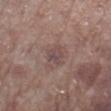Part of a total-body skin-imaging series; this lesion was reviewed on a skin check and was not flagged for biopsy. The lesion is on the right lower leg. This image is a 15 mm lesion crop taken from a total-body photograph. The total-body-photography lesion software estimated a footprint of about 4 mm² and a shape-asymmetry score of about 0.3 (0 = symmetric). The software also gave a lesion color around L≈46 a*≈17 b*≈17 in CIELAB, a lesion–skin lightness drop of about 7, and a lesion-to-skin contrast of about 6 (normalized; higher = more distinct). The analysis additionally found a border-irregularity rating of about 3/10, a within-lesion color-variation index near 2.5/10, and radial color variation of about 1. And it measured a classifier nevus-likeness of about 0/100 and a detector confidence of about 100 out of 100 that the crop contains a lesion. Captured under white-light illumination. The subject is a male aged 68–72.